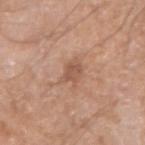workup = catalogued during a skin exam; not biopsied
TBP lesion metrics = a mean CIELAB color near L≈54 a*≈21 b*≈30, a lesion–skin lightness drop of about 9, and a normalized border contrast of about 6; a border-irregularity index near 3/10, internal color variation of about 2 on a 0–10 scale, and peripheral color asymmetry of about 0.5
acquisition = total-body-photography crop, ~15 mm field of view
site = the right upper arm
subject = male, aged approximately 55